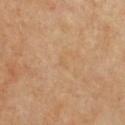| key | value |
|---|---|
| follow-up | no biopsy performed (imaged during a skin exam) |
| automated lesion analysis | a within-lesion color-variation index near 0/10 and peripheral color asymmetry of about 0; a nevus-likeness score of about 0/100 and a lesion-detection confidence of about 100/100 |
| image source | ~15 mm tile from a whole-body skin photo |
| size | about 1 mm |
| illumination | cross-polarized |
| patient | female, roughly 55 years of age |
| location | the chest |